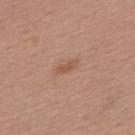The lesion was tiled from a total-body skin photograph and was not biopsied.